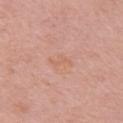Located on the right upper arm.
Captured under white-light illumination.
Measured at roughly 2.5 mm in maximum diameter.
The total-body-photography lesion software estimated roughly 5 lightness units darker than nearby skin. The analysis additionally found an automated nevus-likeness rating near 0 out of 100 and lesion-presence confidence of about 100/100.
A female patient, roughly 50 years of age.
A roughly 15 mm field-of-view crop from a total-body skin photograph.